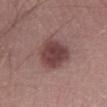{
  "biopsy_status": "not biopsied; imaged during a skin examination",
  "lesion_size": {
    "long_diameter_mm_approx": 4.5
  },
  "image": {
    "source": "total-body photography crop",
    "field_of_view_mm": 15
  },
  "site": "left lower leg",
  "patient": {
    "sex": "male",
    "age_approx": 20
  }
}